The lesion is on the left thigh · this image is a 15 mm lesion crop taken from a total-body photograph · a female subject aged 53–57.
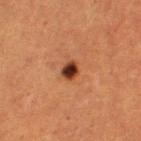Notes:
- diagnosis: a dysplastic (Clark) nevus